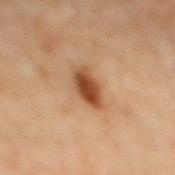follow-up: catalogued during a skin exam; not biopsied | subject: male, approximately 60 years of age | diameter: ~4 mm (longest diameter) | anatomic site: the mid back | imaging modality: ~15 mm crop, total-body skin-cancer survey | automated metrics: a lesion area of about 6.5 mm² and an eccentricity of roughly 0.85; a border-irregularity index near 2.5/10, internal color variation of about 4.5 on a 0–10 scale, and peripheral color asymmetry of about 1.5; a nevus-likeness score of about 100/100.